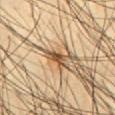| field | value |
|---|---|
| biopsy status | imaged on a skin check; not biopsied |
| anatomic site | the chest |
| lesion diameter | ~2.5 mm (longest diameter) |
| image | ~15 mm crop, total-body skin-cancer survey |
| patient | male, aged 38 to 42 |
| tile lighting | cross-polarized |